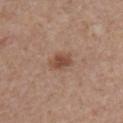Imaged during a routine full-body skin examination; the lesion was not biopsied and no histopathology is available. Imaged with white-light lighting. The lesion's longest dimension is about 3 mm. This image is a 15 mm lesion crop taken from a total-body photograph. The lesion is located on the chest. The subject is a male aged 53–57.